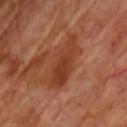Recorded during total-body skin imaging; not selected for excision or biopsy. A region of skin cropped from a whole-body photographic capture, roughly 15 mm wide. A male patient roughly 65 years of age.